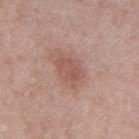follow-up = imaged on a skin check; not biopsied | patient = male, roughly 45 years of age | anatomic site = the left forearm | diameter = about 4 mm | tile lighting = white-light illumination | image-analysis metrics = a lesion area of about 7.5 mm², an eccentricity of roughly 0.7, and two-axis asymmetry of about 0.25; a mean CIELAB color near L≈54 a*≈21 b*≈25 and roughly 8 lightness units darker than nearby skin; a within-lesion color-variation index near 2/10 and peripheral color asymmetry of about 1; an automated nevus-likeness rating near 15 out of 100 and lesion-presence confidence of about 100/100 | acquisition = ~15 mm tile from a whole-body skin photo.